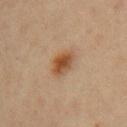follow-up = catalogued during a skin exam; not biopsied | imaging modality = ~15 mm crop, total-body skin-cancer survey | subject = female, aged 43–47 | anatomic site = the left upper arm.